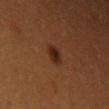The lesion was tiled from a total-body skin photograph and was not biopsied.
The lesion is on the left upper arm.
A female patient aged around 30.
Measured at roughly 2.5 mm in maximum diameter.
The tile uses cross-polarized illumination.
The total-body-photography lesion software estimated an area of roughly 4.5 mm² and a shape eccentricity near 0.6. It also reported an average lesion color of about L≈24 a*≈19 b*≈26 (CIELAB), a lesion–skin lightness drop of about 8, and a normalized border contrast of about 9. And it measured a classifier nevus-likeness of about 95/100 and a detector confidence of about 100 out of 100 that the crop contains a lesion.
A lesion tile, about 15 mm wide, cut from a 3D total-body photograph.